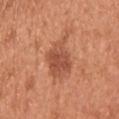Clinical impression: No biopsy was performed on this lesion — it was imaged during a full skin examination and was not determined to be concerning. Context: Measured at roughly 5 mm in maximum diameter. This is a white-light tile. Located on the chest. A male patient, approximately 55 years of age. A roughly 15 mm field-of-view crop from a total-body skin photograph. The lesion-visualizer software estimated an area of roughly 13 mm², an eccentricity of roughly 0.8, and a shape-asymmetry score of about 0.25 (0 = symmetric). And it measured an average lesion color of about L≈52 a*≈27 b*≈34 (CIELAB), about 10 CIELAB-L* units darker than the surrounding skin, and a normalized border contrast of about 7. And it measured border irregularity of about 3.5 on a 0–10 scale, a color-variation rating of about 3.5/10, and a peripheral color-asymmetry measure near 1.